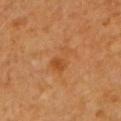Assessment: Part of a total-body skin-imaging series; this lesion was reviewed on a skin check and was not flagged for biopsy. Acquisition and patient details: The tile uses cross-polarized illumination. About 3.5 mm across. The patient is a male aged 58 to 62. A roughly 15 mm field-of-view crop from a total-body skin photograph. The lesion is located on the left upper arm.